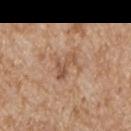| feature | finding |
|---|---|
| lighting | white-light |
| patient | male, in their mid- to late 60s |
| site | the arm |
| lesion size | about 4 mm |
| acquisition | ~15 mm crop, total-body skin-cancer survey |
| TBP lesion metrics | a lesion area of about 5 mm², an outline eccentricity of about 0.85 (0 = round, 1 = elongated), and a symmetry-axis asymmetry near 0.5; a border-irregularity rating of about 6.5/10 and a peripheral color-asymmetry measure near 0.5 |